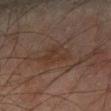Imaged during a routine full-body skin examination; the lesion was not biopsied and no histopathology is available. Cropped from a total-body skin-imaging series; the visible field is about 15 mm. The subject is a male aged 43 to 47. On the left forearm. Automated tile analysis of the lesion measured an automated nevus-likeness rating near 0 out of 100 and a lesion-detection confidence of about 100/100. Imaged with cross-polarized lighting. About 4 mm across.